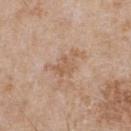Background:
A male subject approximately 65 years of age. A lesion tile, about 15 mm wide, cut from a 3D total-body photograph. Imaged with white-light lighting. From the chest.